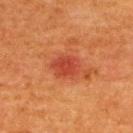The lesion was photographed on a routine skin check and not biopsied; there is no pathology result. On the upper back. A female patient aged approximately 40. The lesion's longest dimension is about 3.5 mm. The lesion-visualizer software estimated a footprint of about 8 mm², an outline eccentricity of about 0.6 (0 = round, 1 = elongated), and a shape-asymmetry score of about 0.15 (0 = symmetric). A roughly 15 mm field-of-view crop from a total-body skin photograph. Captured under cross-polarized illumination.